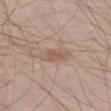<record>
  <biopsy_status>not biopsied; imaged during a skin examination</biopsy_status>
  <patient>
    <sex>male</sex>
    <age_approx>35</age_approx>
  </patient>
  <lesion_size>
    <long_diameter_mm_approx>2.5</long_diameter_mm_approx>
  </lesion_size>
  <lighting>white-light</lighting>
  <site>left thigh</site>
  <image>
    <source>total-body photography crop</source>
    <field_of_view_mm>15</field_of_view_mm>
  </image>
</record>